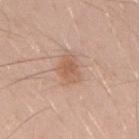The lesion is located on the mid back. Cropped from a total-body skin-imaging series; the visible field is about 15 mm. The patient is a male aged approximately 30.